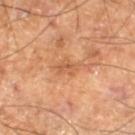workup: no biopsy performed (imaged during a skin exam)
body site: the leg
imaging modality: ~15 mm crop, total-body skin-cancer survey
image-analysis metrics: border irregularity of about 3.5 on a 0–10 scale, a color-variation rating of about 3/10, and peripheral color asymmetry of about 1
tile lighting: cross-polarized
subject: male, aged 68–72
diameter: ~3 mm (longest diameter)